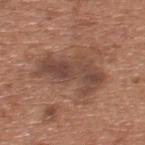Clinical impression:
The lesion was photographed on a routine skin check and not biopsied; there is no pathology result.
Context:
A 15 mm close-up tile from a total-body photography series done for melanoma screening. Located on the upper back. An algorithmic analysis of the crop reported a border-irregularity rating of about 5.5/10, internal color variation of about 4.5 on a 0–10 scale, and radial color variation of about 1.5. It also reported a detector confidence of about 100 out of 100 that the crop contains a lesion. About 7.5 mm across. A male subject, aged approximately 65. Captured under white-light illumination.